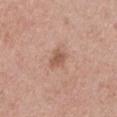notes = total-body-photography surveillance lesion; no biopsy
location = the chest
diameter = ≈3 mm
image-analysis metrics = a lesion area of about 4.5 mm² and a symmetry-axis asymmetry near 0.25; an average lesion color of about L≈56 a*≈21 b*≈29 (CIELAB) and a normalized lesion–skin contrast near 6.5; a color-variation rating of about 2.5/10 and radial color variation of about 1; an automated nevus-likeness rating near 35 out of 100 and lesion-presence confidence of about 100/100
image = ~15 mm crop, total-body skin-cancer survey
patient = male, aged around 60